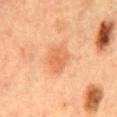location: the abdomen | patient: female, aged around 60 | image: total-body-photography crop, ~15 mm field of view | lighting: cross-polarized illumination.